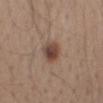Part of a total-body skin-imaging series; this lesion was reviewed on a skin check and was not flagged for biopsy.